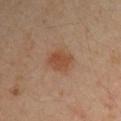biopsy status — imaged on a skin check; not biopsied | image source — ~15 mm tile from a whole-body skin photo | patient — male, aged 38 to 42 | anatomic site — the right upper arm | automated metrics — an area of roughly 7 mm² and an outline eccentricity of about 0.6 (0 = round, 1 = elongated); a lesion color around L≈46 a*≈21 b*≈31 in CIELAB and a normalized border contrast of about 7; an automated nevus-likeness rating near 95 out of 100.